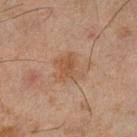No biopsy was performed on this lesion — it was imaged during a full skin examination and was not determined to be concerning.
A male patient aged around 45.
Approximately 4 mm at its widest.
This image is a 15 mm lesion crop taken from a total-body photograph.
Imaged with cross-polarized lighting.
From the left lower leg.